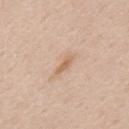Q: Is there a histopathology result?
A: no biopsy performed (imaged during a skin exam)
Q: What are the patient's age and sex?
A: male, aged around 60
Q: What is the anatomic site?
A: the mid back
Q: Automated lesion metrics?
A: border irregularity of about 3 on a 0–10 scale, internal color variation of about 0 on a 0–10 scale, and radial color variation of about 0
Q: How was this image acquired?
A: ~15 mm crop, total-body skin-cancer survey
Q: Illumination type?
A: white-light